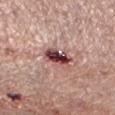The lesion is located on the left lower leg. The patient is a male aged 48–52. A lesion tile, about 15 mm wide, cut from a 3D total-body photograph. Captured under white-light illumination.